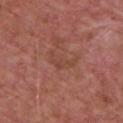| feature | finding |
|---|---|
| diameter | about 3.5 mm |
| patient | male, aged 73 to 77 |
| image source | ~15 mm tile from a whole-body skin photo |
| TBP lesion metrics | a symmetry-axis asymmetry near 0.55; an average lesion color of about L≈45 a*≈24 b*≈28 (CIELAB) and a normalized border contrast of about 4.5 |
| site | the chest |
| tile lighting | white-light illumination |